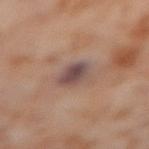Clinical impression: The lesion was tiled from a total-body skin photograph and was not biopsied. Context: From the right thigh. A female patient about 55 years old. This image is a 15 mm lesion crop taken from a total-body photograph. The recorded lesion diameter is about 3.5 mm. The tile uses cross-polarized illumination.